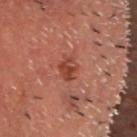Clinical impression: No biopsy was performed on this lesion — it was imaged during a full skin examination and was not determined to be concerning. Background: Imaged with cross-polarized lighting. A 15 mm close-up tile from a total-body photography series done for melanoma screening. An algorithmic analysis of the crop reported a shape-asymmetry score of about 0.3 (0 = symmetric). The analysis additionally found a border-irregularity index near 2.5/10 and peripheral color asymmetry of about 1. It also reported an automated nevus-likeness rating near 45 out of 100 and lesion-presence confidence of about 100/100. From the head or neck. About 2.5 mm across. The patient is a male roughly 60 years of age.